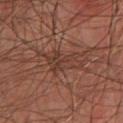Recorded during total-body skin imaging; not selected for excision or biopsy.
From the chest.
The tile uses cross-polarized illumination.
A male patient, approximately 65 years of age.
Automated image analysis of the tile measured a footprint of about 10 mm² and a symmetry-axis asymmetry near 0.5.
A close-up tile cropped from a whole-body skin photograph, about 15 mm across.